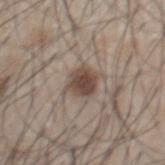This lesion was catalogued during total-body skin photography and was not selected for biopsy.
The patient is a male aged approximately 45.
From the chest.
The recorded lesion diameter is about 3 mm.
A 15 mm close-up tile from a total-body photography series done for melanoma screening.
An algorithmic analysis of the crop reported an area of roughly 8 mm², an eccentricity of roughly 0.35, and a symmetry-axis asymmetry near 0.15. It also reported a nevus-likeness score of about 95/100 and lesion-presence confidence of about 100/100.